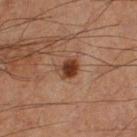Image and clinical context: Longest diameter approximately 2.5 mm. The lesion is on the left thigh. A region of skin cropped from a whole-body photographic capture, roughly 15 mm wide. Imaged with cross-polarized lighting. An algorithmic analysis of the crop reported a mean CIELAB color near L≈35 a*≈21 b*≈28, about 12 CIELAB-L* units darker than the surrounding skin, and a lesion-to-skin contrast of about 10.5 (normalized; higher = more distinct). The software also gave a border-irregularity rating of about 2.5/10, a within-lesion color-variation index near 4.5/10, and radial color variation of about 1. A male subject in their 60s.